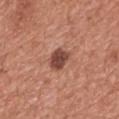<tbp_lesion>
<lesion_size>
  <long_diameter_mm_approx>2.5</long_diameter_mm_approx>
</lesion_size>
<image>
  <source>total-body photography crop</source>
  <field_of_view_mm>15</field_of_view_mm>
</image>
<site>chest</site>
<patient>
  <sex>male</sex>
  <age_approx>45</age_approx>
</patient>
</tbp_lesion>